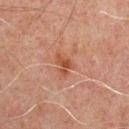Part of a total-body skin-imaging series; this lesion was reviewed on a skin check and was not flagged for biopsy.
Longest diameter approximately 3 mm.
A male patient aged around 60.
A close-up tile cropped from a whole-body skin photograph, about 15 mm across.
This is a cross-polarized tile.
The lesion is located on the chest.